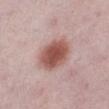Impression: The lesion was photographed on a routine skin check and not biopsied; there is no pathology result. Background: Located on the left lower leg. The lesion-visualizer software estimated a nevus-likeness score of about 100/100 and lesion-presence confidence of about 100/100. A roughly 15 mm field-of-view crop from a total-body skin photograph. The patient is a female approximately 45 years of age. Imaged with white-light lighting.